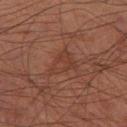The lesion was tiled from a total-body skin photograph and was not biopsied.
About 3.5 mm across.
Captured under cross-polarized illumination.
Cropped from a total-body skin-imaging series; the visible field is about 15 mm.
The lesion is located on the left thigh.
A male patient aged around 70.
The lesion-visualizer software estimated an eccentricity of roughly 0.55. It also reported a border-irregularity index near 4.5/10 and a color-variation rating of about 2.5/10.